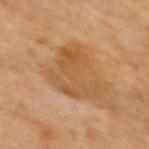Part of a total-body skin-imaging series; this lesion was reviewed on a skin check and was not flagged for biopsy. Located on the upper back. Imaged with cross-polarized lighting. A female subject, aged approximately 70. The lesion's longest dimension is about 5.5 mm. A 15 mm crop from a total-body photograph taken for skin-cancer surveillance.